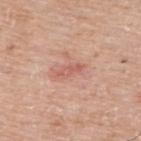Q: Was a biopsy performed?
A: no biopsy performed (imaged during a skin exam)
Q: How was the tile lit?
A: white-light
Q: What is the imaging modality?
A: ~15 mm crop, total-body skin-cancer survey
Q: What is the anatomic site?
A: the upper back
Q: Who is the patient?
A: male, about 75 years old